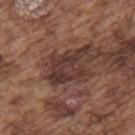Background: A male patient aged 73–77. Imaged with white-light lighting. A 15 mm close-up extracted from a 3D total-body photography capture. The lesion-visualizer software estimated a footprint of about 12 mm², an eccentricity of roughly 0.8, and two-axis asymmetry of about 0.45. It also reported a nevus-likeness score of about 0/100 and a detector confidence of about 90 out of 100 that the crop contains a lesion. On the upper back. About 6 mm across.